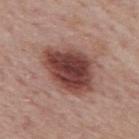Notes:
– body site · the mid back
– lesion diameter · ≈7 mm
– image source · 15 mm crop, total-body photography
– subject · male, in their mid-70s
– lighting · white-light illumination